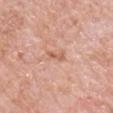Case summary:
- follow-up · catalogued during a skin exam; not biopsied
- patient · male, in their 60s
- image · ~15 mm crop, total-body skin-cancer survey
- anatomic site · the right upper arm